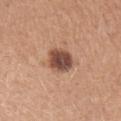The lesion was photographed on a routine skin check and not biopsied; there is no pathology result. On the left forearm. A female patient, about 30 years old. Longest diameter approximately 3.5 mm. Captured under white-light illumination. Cropped from a whole-body photographic skin survey; the tile spans about 15 mm.